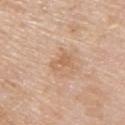follow-up = total-body-photography surveillance lesion; no biopsy
location = the upper back
acquisition = total-body-photography crop, ~15 mm field of view
subject = male, aged 78 to 82